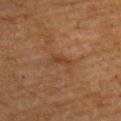Q: Is there a histopathology result?
A: catalogued during a skin exam; not biopsied
Q: What is the imaging modality?
A: ~15 mm crop, total-body skin-cancer survey
Q: What is the anatomic site?
A: the left upper arm
Q: Illumination type?
A: cross-polarized illumination
Q: Automated lesion metrics?
A: a lesion–skin lightness drop of about 6 and a normalized border contrast of about 5.5
Q: How large is the lesion?
A: about 2.5 mm
Q: What are the patient's age and sex?
A: female, roughly 55 years of age